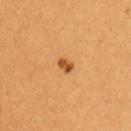The lesion-visualizer software estimated an area of roughly 2.5 mm², an eccentricity of roughly 0.7, and two-axis asymmetry of about 0.2. It also reported a detector confidence of about 100 out of 100 that the crop contains a lesion. From the left upper arm. A female patient, aged approximately 30. The tile uses cross-polarized illumination. Measured at roughly 2 mm in maximum diameter. A close-up tile cropped from a whole-body skin photograph, about 15 mm across.